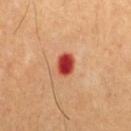Recorded during total-body skin imaging; not selected for excision or biopsy. Cropped from a total-body skin-imaging series; the visible field is about 15 mm. From the chest. The lesion-visualizer software estimated a lesion area of about 5.5 mm², an eccentricity of roughly 0.7, and a symmetry-axis asymmetry near 0.2. It also reported a mean CIELAB color near L≈44 a*≈38 b*≈34, about 18 CIELAB-L* units darker than the surrounding skin, and a lesion-to-skin contrast of about 12.5 (normalized; higher = more distinct). The software also gave a border-irregularity rating of about 1.5/10 and peripheral color asymmetry of about 1. Approximately 3 mm at its widest. This is a cross-polarized tile. A male subject, roughly 60 years of age.